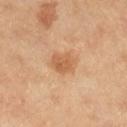Impression:
The lesion was tiled from a total-body skin photograph and was not biopsied.
Image and clinical context:
A female patient, roughly 60 years of age. A lesion tile, about 15 mm wide, cut from a 3D total-body photograph. The tile uses cross-polarized illumination. On the right lower leg. About 3 mm across.